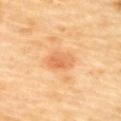<record>
  <lighting>cross-polarized</lighting>
  <automated_metrics>
    <border_irregularity_0_10>2.0</border_irregularity_0_10>
    <peripheral_color_asymmetry>2.0</peripheral_color_asymmetry>
    <nevus_likeness_0_100>35</nevus_likeness_0_100>
    <lesion_detection_confidence_0_100>100</lesion_detection_confidence_0_100>
  </automated_metrics>
  <site>upper back</site>
  <patient>
    <sex>female</sex>
    <age_approx>60</age_approx>
  </patient>
  <image>
    <source>total-body photography crop</source>
    <field_of_view_mm>15</field_of_view_mm>
  </image>
</record>